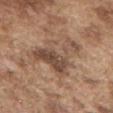Assessment: Recorded during total-body skin imaging; not selected for excision or biopsy. Clinical summary: A male patient, about 75 years old. From the chest. Cropped from a total-body skin-imaging series; the visible field is about 15 mm.